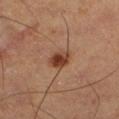workup: no biopsy performed (imaged during a skin exam); body site: the left lower leg; lesion diameter: ≈2.5 mm; patient: male, in their 60s; illumination: cross-polarized illumination; acquisition: ~15 mm crop, total-body skin-cancer survey.